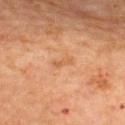The lesion was photographed on a routine skin check and not biopsied; there is no pathology result.
Approximately 2.5 mm at its widest.
A 15 mm close-up extracted from a 3D total-body photography capture.
The tile uses cross-polarized illumination.
The lesion is located on the upper back.
The subject is a female aged around 70.